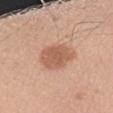<lesion>
<biopsy_status>not biopsied; imaged during a skin examination</biopsy_status>
<lighting>white-light</lighting>
<image>
  <source>total-body photography crop</source>
  <field_of_view_mm>15</field_of_view_mm>
</image>
<automated_metrics>
  <area_mm2_approx>11.0</area_mm2_approx>
  <eccentricity>0.3</eccentricity>
  <shape_asymmetry>0.15</shape_asymmetry>
  <border_irregularity_0_10>1.5</border_irregularity_0_10>
  <color_variation_0_10>2.5</color_variation_0_10>
  <peripheral_color_asymmetry>0.5</peripheral_color_asymmetry>
</automated_metrics>
<lesion_size>
  <long_diameter_mm_approx>4.0</long_diameter_mm_approx>
</lesion_size>
<site>left forearm</site>
<patient>
  <sex>female</sex>
  <age_approx>25</age_approx>
</patient>
</lesion>